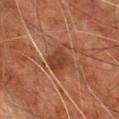Q: Was this lesion biopsied?
A: total-body-photography surveillance lesion; no biopsy
Q: What kind of image is this?
A: total-body-photography crop, ~15 mm field of view
Q: Where on the body is the lesion?
A: the chest
Q: What are the patient's age and sex?
A: male, approximately 65 years of age